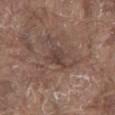Notes:
* workup — imaged on a skin check; not biopsied
* TBP lesion metrics — a footprint of about 4.5 mm², a shape eccentricity near 0.7, and a symmetry-axis asymmetry near 0.35; an average lesion color of about L≈41 a*≈16 b*≈22 (CIELAB) and about 7 CIELAB-L* units darker than the surrounding skin; a within-lesion color-variation index near 2.5/10 and radial color variation of about 1; a nevus-likeness score of about 5/100 and a detector confidence of about 90 out of 100 that the crop contains a lesion
* lighting — white-light
* location — the abdomen
* image — 15 mm crop, total-body photography
* diameter — ≈3 mm
* patient — male, roughly 80 years of age